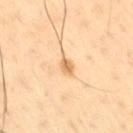Clinical impression:
The lesion was photographed on a routine skin check and not biopsied; there is no pathology result.
Context:
A male patient, in their mid- to late 50s. The total-body-photography lesion software estimated a footprint of about 2.5 mm² and a shape eccentricity near 0.6. And it measured lesion-presence confidence of about 100/100. The lesion's longest dimension is about 2 mm. Captured under cross-polarized illumination. A 15 mm close-up tile from a total-body photography series done for melanoma screening. The lesion is located on the right thigh.